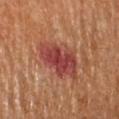Q: How was this image acquired?
A: total-body-photography crop, ~15 mm field of view
Q: What are the patient's age and sex?
A: female, about 65 years old
Q: What is the anatomic site?
A: the left arm
Q: What lighting was used for the tile?
A: cross-polarized